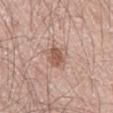Clinical impression:
Part of a total-body skin-imaging series; this lesion was reviewed on a skin check and was not flagged for biopsy.
Context:
Automated tile analysis of the lesion measured a classifier nevus-likeness of about 80/100. The tile uses white-light illumination. From the abdomen. A male subject, aged 63 to 67. The recorded lesion diameter is about 3 mm. A 15 mm close-up tile from a total-body photography series done for melanoma screening.